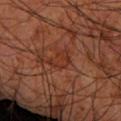Clinical impression: Part of a total-body skin-imaging series; this lesion was reviewed on a skin check and was not flagged for biopsy. Context: The lesion is on the left forearm. A male subject approximately 60 years of age. The recorded lesion diameter is about 3.5 mm. An algorithmic analysis of the crop reported a shape eccentricity near 0.8 and a symmetry-axis asymmetry near 0.4. It also reported an average lesion color of about L≈32 a*≈25 b*≈30 (CIELAB) and a normalized lesion–skin contrast near 5.5. It also reported border irregularity of about 3.5 on a 0–10 scale, a within-lesion color-variation index near 2/10, and radial color variation of about 0.5. It also reported a nevus-likeness score of about 0/100. A roughly 15 mm field-of-view crop from a total-body skin photograph.